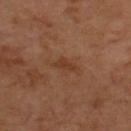Impression: Imaged during a routine full-body skin examination; the lesion was not biopsied and no histopathology is available. Clinical summary: The subject is aged 63 to 67. This is a cross-polarized tile. The lesion is located on the back. Approximately 2.5 mm at its widest. Automated image analysis of the tile measured an area of roughly 3 mm² and a symmetry-axis asymmetry near 0.45. The analysis additionally found a lesion color around L≈37 a*≈21 b*≈31 in CIELAB and roughly 6 lightness units darker than nearby skin. It also reported a border-irregularity rating of about 4.5/10, a color-variation rating of about 0.5/10, and peripheral color asymmetry of about 0. The analysis additionally found lesion-presence confidence of about 100/100. Cropped from a total-body skin-imaging series; the visible field is about 15 mm.